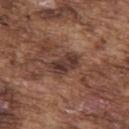{
  "biopsy_status": "not biopsied; imaged during a skin examination",
  "image": {
    "source": "total-body photography crop",
    "field_of_view_mm": 15
  },
  "lighting": "white-light",
  "automated_metrics": {
    "area_mm2_approx": 7.0,
    "eccentricity": 0.85,
    "shape_asymmetry": 0.35,
    "border_irregularity_0_10": 3.5,
    "color_variation_0_10": 4.5,
    "peripheral_color_asymmetry": 1.5,
    "lesion_detection_confidence_0_100": 95
  },
  "patient": {
    "sex": "male",
    "age_approx": 75
  },
  "site": "upper back"
}